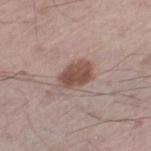This lesion was catalogued during total-body skin photography and was not selected for biopsy.